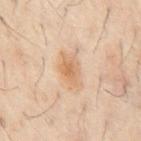Longest diameter approximately 4.5 mm.
A 15 mm close-up extracted from a 3D total-body photography capture.
The lesion-visualizer software estimated a lesion area of about 9 mm². The analysis additionally found a border-irregularity index near 2.5/10, a within-lesion color-variation index near 4/10, and radial color variation of about 1. The software also gave a nevus-likeness score of about 25/100.
A male subject aged approximately 60.
Imaged with cross-polarized lighting.
The lesion is on the abdomen.